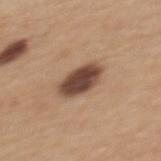The lesion was tiled from a total-body skin photograph and was not biopsied. The lesion is on the upper back. Longest diameter approximately 5 mm. Cropped from a total-body skin-imaging series; the visible field is about 15 mm. Automated tile analysis of the lesion measured a nevus-likeness score of about 65/100 and lesion-presence confidence of about 100/100. This is a white-light tile. A female patient, approximately 30 years of age.